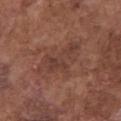Q: Is there a histopathology result?
A: no biopsy performed (imaged during a skin exam)
Q: What is the lesion's diameter?
A: ≈6.5 mm
Q: Patient demographics?
A: male, in their mid-70s
Q: Lesion location?
A: the front of the torso
Q: What is the imaging modality?
A: ~15 mm tile from a whole-body skin photo